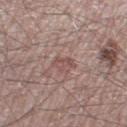Clinical summary:
The patient is a male aged 48 to 52. The lesion is on the right thigh. This image is a 15 mm lesion crop taken from a total-body photograph.